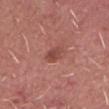notes: imaged on a skin check; not biopsied
site: the head or neck
image-analysis metrics: a border-irregularity index near 2.5/10, a within-lesion color-variation index near 2.5/10, and radial color variation of about 1
subject: male, aged around 60
image: ~15 mm tile from a whole-body skin photo
lighting: white-light